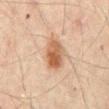The lesion was photographed on a routine skin check and not biopsied; there is no pathology result.
A 15 mm crop from a total-body photograph taken for skin-cancer surveillance.
Approximately 4 mm at its widest.
A male patient aged 43 to 47.
The tile uses cross-polarized illumination.
From the front of the torso.